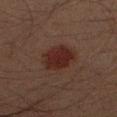notes: no biopsy performed (imaged during a skin exam) | subject: male, roughly 45 years of age | image: total-body-photography crop, ~15 mm field of view | body site: the right forearm | lighting: cross-polarized illumination.